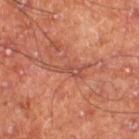Imaged during a routine full-body skin examination; the lesion was not biopsied and no histopathology is available.
This is a cross-polarized tile.
The lesion is located on the right thigh.
A male patient, approximately 60 years of age.
An algorithmic analysis of the crop reported an average lesion color of about L≈46 a*≈25 b*≈28 (CIELAB), a lesion–skin lightness drop of about 7, and a normalized border contrast of about 5.5. It also reported a border-irregularity rating of about 10/10, a within-lesion color-variation index near 1/10, and peripheral color asymmetry of about 0.
A region of skin cropped from a whole-body photographic capture, roughly 15 mm wide.
Measured at roughly 5 mm in maximum diameter.